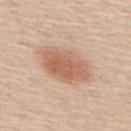Assessment:
Captured during whole-body skin photography for melanoma surveillance; the lesion was not biopsied.
Acquisition and patient details:
The lesion-visualizer software estimated a lesion area of about 19 mm², a shape eccentricity near 0.8, and a symmetry-axis asymmetry near 0.15. The analysis additionally found an average lesion color of about L≈62 a*≈21 b*≈31 (CIELAB), roughly 11 lightness units darker than nearby skin, and a normalized border contrast of about 7.5. The software also gave a color-variation rating of about 4/10 and radial color variation of about 1. And it measured an automated nevus-likeness rating near 95 out of 100 and lesion-presence confidence of about 100/100. A roughly 15 mm field-of-view crop from a total-body skin photograph. Measured at roughly 7 mm in maximum diameter. This is a white-light tile. The subject is a male about 60 years old. The lesion is on the back.